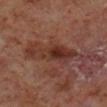<tbp_lesion>
<biopsy_status>not biopsied; imaged during a skin examination</biopsy_status>
<lighting>cross-polarized</lighting>
<automated_metrics>
  <cielab_L>30</cielab_L>
  <cielab_a>21</cielab_a>
  <cielab_b>24</cielab_b>
  <vs_skin_darker_L>9.0</vs_skin_darker_L>
  <border_irregularity_0_10>6.5</border_irregularity_0_10>
  <color_variation_0_10>5.5</color_variation_0_10>
  <peripheral_color_asymmetry>1.5</peripheral_color_asymmetry>
  <nevus_likeness_0_100>0</nevus_likeness_0_100>
</automated_metrics>
<lesion_size>
  <long_diameter_mm_approx>7.0</long_diameter_mm_approx>
</lesion_size>
<site>right lower leg</site>
<image>
  <source>total-body photography crop</source>
  <field_of_view_mm>15</field_of_view_mm>
</image>
<patient>
  <sex>male</sex>
  <age_approx>60</age_approx>
</patient>
</tbp_lesion>